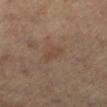Notes:
• workup · catalogued during a skin exam; not biopsied